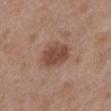{"biopsy_status": "not biopsied; imaged during a skin examination", "site": "mid back", "automated_metrics": {"area_mm2_approx": 11.0, "eccentricity": 0.65, "cielab_L": 47, "cielab_a": 20, "cielab_b": 27, "vs_skin_darker_L": 11.0, "vs_skin_contrast_norm": 8.0}, "image": {"source": "total-body photography crop", "field_of_view_mm": 15}, "patient": {"sex": "male", "age_approx": 50}, "lighting": "white-light", "lesion_size": {"long_diameter_mm_approx": 4.0}}